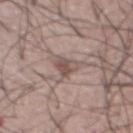Clinical impression: Part of a total-body skin-imaging series; this lesion was reviewed on a skin check and was not flagged for biopsy. Clinical summary: A male patient aged around 55. Automated image analysis of the tile measured a lesion area of about 5 mm². The analysis additionally found a nevus-likeness score of about 0/100 and a detector confidence of about 85 out of 100 that the crop contains a lesion. This is a white-light tile. The lesion is on the abdomen. A 15 mm crop from a total-body photograph taken for skin-cancer surveillance.